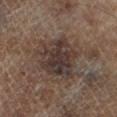biopsy status: total-body-photography surveillance lesion; no biopsy
subject: male, aged around 65
acquisition: ~15 mm tile from a whole-body skin photo
location: the left lower leg
automated metrics: an area of roughly 19 mm², an eccentricity of roughly 0.5, and a shape-asymmetry score of about 0.25 (0 = symmetric); a border-irregularity index near 3.5/10, a color-variation rating of about 5.5/10, and peripheral color asymmetry of about 2; a nevus-likeness score of about 0/100 and a detector confidence of about 95 out of 100 that the crop contains a lesion
lesion diameter: about 5.5 mm
illumination: cross-polarized illumination